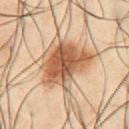The lesion was photographed on a routine skin check and not biopsied; there is no pathology result.
The lesion's longest dimension is about 6 mm.
A close-up tile cropped from a whole-body skin photograph, about 15 mm across.
Automated tile analysis of the lesion measured a lesion area of about 22 mm², a shape eccentricity near 0.7, and a shape-asymmetry score of about 0.3 (0 = symmetric). It also reported an average lesion color of about L≈43 a*≈17 b*≈28 (CIELAB), roughly 13 lightness units darker than nearby skin, and a lesion-to-skin contrast of about 10 (normalized; higher = more distinct). The software also gave a border-irregularity index near 3.5/10, a within-lesion color-variation index near 5/10, and peripheral color asymmetry of about 1.5. And it measured a classifier nevus-likeness of about 95/100 and lesion-presence confidence of about 100/100.
From the abdomen.
The tile uses cross-polarized illumination.
A male subject, aged 48–52.